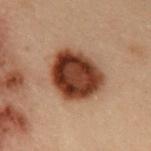Case summary:
• biopsy status — total-body-photography surveillance lesion; no biopsy
• patient — male, in their mid-50s
• image — total-body-photography crop, ~15 mm field of view
• site — the front of the torso